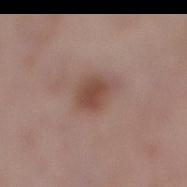The recorded lesion diameter is about 3.5 mm. The lesion is located on the right lower leg. The tile uses white-light illumination. Cropped from a total-body skin-imaging series; the visible field is about 15 mm. A female subject, aged approximately 65.